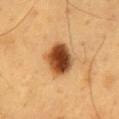Cropped from a whole-body photographic skin survey; the tile spans about 15 mm. About 4 mm across. The lesion is on the mid back. The subject is a male about 60 years old.